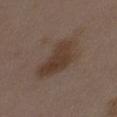Background: A region of skin cropped from a whole-body photographic capture, roughly 15 mm wide. Captured under white-light illumination. About 6.5 mm across. From the abdomen. An algorithmic analysis of the crop reported a mean CIELAB color near L≈38 a*≈14 b*≈24, a lesion–skin lightness drop of about 8, and a normalized lesion–skin contrast near 8. The analysis additionally found a detector confidence of about 100 out of 100 that the crop contains a lesion. A female subject, in their mid-30s.